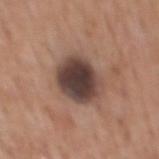Background:
An algorithmic analysis of the crop reported a footprint of about 16 mm², a shape eccentricity near 0.45, and a shape-asymmetry score of about 0.15 (0 = symmetric). The software also gave a border-irregularity rating of about 1.5/10, internal color variation of about 5.5 on a 0–10 scale, and a peripheral color-asymmetry measure near 1.5. A lesion tile, about 15 mm wide, cut from a 3D total-body photograph. Imaged with white-light lighting. Longest diameter approximately 4.5 mm. On the mid back. The patient is a male in their 60s.